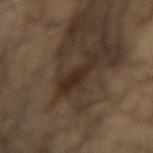| key | value |
|---|---|
| follow-up | total-body-photography surveillance lesion; no biopsy |
| anatomic site | the leg |
| image | total-body-photography crop, ~15 mm field of view |
| subject | male, aged around 50 |
| lighting | cross-polarized |
| automated lesion analysis | an eccentricity of roughly 0.95 and a symmetry-axis asymmetry near 0.35; a mean CIELAB color near L≈19 a*≈10 b*≈17 and a normalized border contrast of about 9.5; a border-irregularity index near 5/10 and a peripheral color-asymmetry measure near 0.5; a nevus-likeness score of about 10/100 |